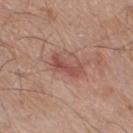image: ~15 mm crop, total-body skin-cancer survey | location: the right thigh | automated lesion analysis: a footprint of about 6.5 mm² and a shape eccentricity near 0.85; a border-irregularity rating of about 5/10, internal color variation of about 3 on a 0–10 scale, and peripheral color asymmetry of about 1 | lighting: white-light | lesion size: ≈4 mm | subject: male, about 80 years old.